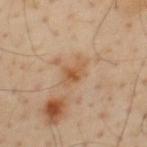Clinical impression: The lesion was photographed on a routine skin check and not biopsied; there is no pathology result. Background: A lesion tile, about 15 mm wide, cut from a 3D total-body photograph. On the back. The patient is a male aged 53–57. This is a cross-polarized tile. Approximately 3 mm at its widest.